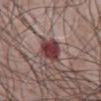Notes:
• biopsy status · imaged on a skin check; not biopsied
• lesion size · about 3.5 mm
• body site · the abdomen
• lighting · white-light
• patient · male, aged 63 to 67
• image source · 15 mm crop, total-body photography
• image-analysis metrics · a lesion area of about 8 mm² and a symmetry-axis asymmetry near 0.2